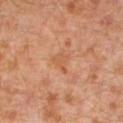notes: total-body-photography surveillance lesion; no biopsy | patient: male, aged approximately 30 | site: the left lower leg | imaging modality: ~15 mm crop, total-body skin-cancer survey.